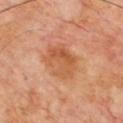Impression: Recorded during total-body skin imaging; not selected for excision or biopsy. Context: Cropped from a whole-body photographic skin survey; the tile spans about 15 mm. About 4.5 mm across. The lesion is located on the front of the torso. Automated tile analysis of the lesion measured an automated nevus-likeness rating near 10 out of 100. A male patient aged around 70.